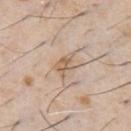No biopsy was performed on this lesion — it was imaged during a full skin examination and was not determined to be concerning.
Cropped from a whole-body photographic skin survey; the tile spans about 15 mm.
The lesion-visualizer software estimated an area of roughly 5 mm² and an eccentricity of roughly 0.75. It also reported border irregularity of about 4 on a 0–10 scale, internal color variation of about 5 on a 0–10 scale, and peripheral color asymmetry of about 2. And it measured a nevus-likeness score of about 5/100 and a lesion-detection confidence of about 100/100.
On the chest.
A male patient, aged around 60.
This is a white-light tile.
Approximately 3.5 mm at its widest.